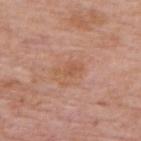Assessment: Recorded during total-body skin imaging; not selected for excision or biopsy. Background: The patient is a male about 75 years old. The lesion is on the upper back. The recorded lesion diameter is about 3 mm. A region of skin cropped from a whole-body photographic capture, roughly 15 mm wide.